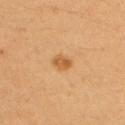Assessment: The lesion was tiled from a total-body skin photograph and was not biopsied.